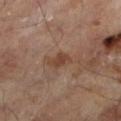notes — imaged on a skin check; not biopsied | site — the left lower leg | automated lesion analysis — a lesion area of about 3.5 mm² and an eccentricity of roughly 0.7; a classifier nevus-likeness of about 5/100 and a detector confidence of about 100 out of 100 that the crop contains a lesion | patient — male, in their mid- to late 60s | image — ~15 mm crop, total-body skin-cancer survey.